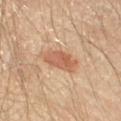notes — total-body-photography surveillance lesion; no biopsy | lesion size — ~3.5 mm (longest diameter) | body site — the right upper arm | imaging modality — 15 mm crop, total-body photography | image-analysis metrics — a lesion area of about 7.5 mm², an eccentricity of roughly 0.7, and a shape-asymmetry score of about 0.2 (0 = symmetric); an average lesion color of about L≈57 a*≈23 b*≈33 (CIELAB) and a normalized lesion–skin contrast near 7; an automated nevus-likeness rating near 100 out of 100 and a lesion-detection confidence of about 100/100 | illumination — cross-polarized illumination | subject — male, aged approximately 65.